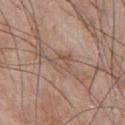A roughly 15 mm field-of-view crop from a total-body skin photograph.
The lesion is located on the chest.
A male subject, aged approximately 55.